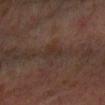follow-up: total-body-photography surveillance lesion; no biopsy.